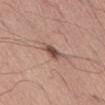This lesion was catalogued during total-body skin photography and was not selected for biopsy. A male patient aged 53–57. Approximately 3 mm at its widest. A region of skin cropped from a whole-body photographic capture, roughly 15 mm wide. Captured under white-light illumination. From the abdomen.